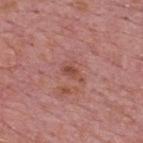workup: no biopsy performed (imaged during a skin exam) | patient: male, aged 73–77 | tile lighting: white-light | lesion size: ≈3 mm | location: the upper back | TBP lesion metrics: a border-irregularity index near 4/10, a within-lesion color-variation index near 2/10, and peripheral color asymmetry of about 1 | imaging modality: 15 mm crop, total-body photography.